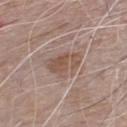Q: Was this lesion biopsied?
A: imaged on a skin check; not biopsied